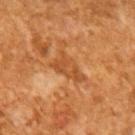Case summary:
• follow-up · imaged on a skin check; not biopsied
• subject · male, approximately 65 years of age
• acquisition · ~15 mm crop, total-body skin-cancer survey
• illumination · cross-polarized
• TBP lesion metrics · a lesion-detection confidence of about 60/100